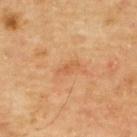biopsy status: imaged on a skin check; not biopsied
lighting: cross-polarized
subject: male, aged approximately 70
image-analysis metrics: an average lesion color of about L≈56 a*≈24 b*≈39 (CIELAB) and roughly 7 lightness units darker than nearby skin; border irregularity of about 4.5 on a 0–10 scale and peripheral color asymmetry of about 0
image: ~15 mm tile from a whole-body skin photo
diameter: ≈2.5 mm
body site: the upper back